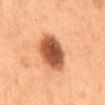No biopsy was performed on this lesion — it was imaged during a full skin examination and was not determined to be concerning.
A male patient approximately 55 years of age.
Automated image analysis of the tile measured a lesion–skin lightness drop of about 18 and a lesion-to-skin contrast of about 12 (normalized; higher = more distinct). The software also gave a color-variation rating of about 6/10 and a peripheral color-asymmetry measure near 1.5. And it measured an automated nevus-likeness rating near 100 out of 100 and a lesion-detection confidence of about 100/100.
This is a cross-polarized tile.
Approximately 5.5 mm at its widest.
Cropped from a whole-body photographic skin survey; the tile spans about 15 mm.
The lesion is on the back.